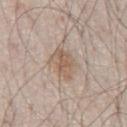<tbp_lesion>
<biopsy_status>not biopsied; imaged during a skin examination</biopsy_status>
<patient>
  <sex>male</sex>
  <age_approx>80</age_approx>
</patient>
<image>
  <source>total-body photography crop</source>
  <field_of_view_mm>15</field_of_view_mm>
</image>
<lesion_size>
  <long_diameter_mm_approx>3.5</long_diameter_mm_approx>
</lesion_size>
<lighting>white-light</lighting>
<site>chest</site>
</tbp_lesion>